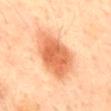The total-body-photography lesion software estimated an outline eccentricity of about 0.7 (0 = round, 1 = elongated). It also reported a mean CIELAB color near L≈68 a*≈30 b*≈43, roughly 15 lightness units darker than nearby skin, and a normalized border contrast of about 9. The analysis additionally found border irregularity of about 2 on a 0–10 scale and internal color variation of about 4.5 on a 0–10 scale.
The lesion is on the mid back.
Cropped from a total-body skin-imaging series; the visible field is about 15 mm.
Measured at roughly 6 mm in maximum diameter.
The subject is a male aged approximately 50.
This is a cross-polarized tile.